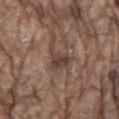This lesion was catalogued during total-body skin photography and was not selected for biopsy.
This is a white-light tile.
The lesion is on the mid back.
A male patient in their 80s.
Longest diameter approximately 3.5 mm.
Automated tile analysis of the lesion measured a lesion area of about 6 mm², a shape eccentricity near 0.7, and a shape-asymmetry score of about 0.55 (0 = symmetric). The analysis additionally found a mean CIELAB color near L≈41 a*≈16 b*≈22 and a lesion–skin lightness drop of about 9. It also reported a border-irregularity rating of about 6.5/10 and a color-variation rating of about 3.5/10. It also reported a classifier nevus-likeness of about 0/100 and a lesion-detection confidence of about 70/100.
A roughly 15 mm field-of-view crop from a total-body skin photograph.